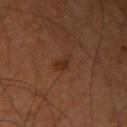This lesion was catalogued during total-body skin photography and was not selected for biopsy. A lesion tile, about 15 mm wide, cut from a 3D total-body photograph. An algorithmic analysis of the crop reported an area of roughly 3 mm² and an outline eccentricity of about 0.85 (0 = round, 1 = elongated). It also reported a within-lesion color-variation index near 1/10. It also reported a detector confidence of about 100 out of 100 that the crop contains a lesion. A male subject, roughly 60 years of age. From the right upper arm. About 3 mm across. Imaged with cross-polarized lighting.